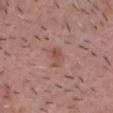biopsy status = catalogued during a skin exam; not biopsied
tile lighting = white-light illumination
subject = male, about 55 years old
imaging modality = ~15 mm crop, total-body skin-cancer survey
anatomic site = the head or neck
lesion diameter = about 3 mm